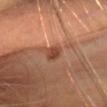No biopsy was performed on this lesion — it was imaged during a full skin examination and was not determined to be concerning. Longest diameter approximately 2.5 mm. A female patient aged 58 to 62. From the head or neck. A region of skin cropped from a whole-body photographic capture, roughly 15 mm wide. This is a cross-polarized tile.